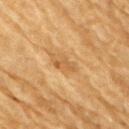| field | value |
|---|---|
| follow-up | catalogued during a skin exam; not biopsied |
| illumination | cross-polarized illumination |
| imaging modality | total-body-photography crop, ~15 mm field of view |
| subject | male, roughly 85 years of age or older |
| anatomic site | the arm |